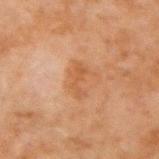Impression: The lesion was tiled from a total-body skin photograph and was not biopsied. Image and clinical context: The lesion is located on the arm. The recorded lesion diameter is about 3.5 mm. A 15 mm crop from a total-body photograph taken for skin-cancer surveillance. Imaged with cross-polarized lighting. A male patient aged 43 to 47. An algorithmic analysis of the crop reported a mean CIELAB color near L≈46 a*≈20 b*≈32, a lesion–skin lightness drop of about 6, and a normalized border contrast of about 5.5. The analysis additionally found a classifier nevus-likeness of about 0/100 and a lesion-detection confidence of about 100/100.